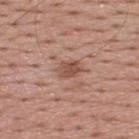The lesion was photographed on a routine skin check and not biopsied; there is no pathology result. A male subject roughly 55 years of age. Approximately 3 mm at its widest. The tile uses white-light illumination. An algorithmic analysis of the crop reported an area of roughly 4.5 mm². The analysis additionally found a mean CIELAB color near L≈51 a*≈22 b*≈27 and about 10 CIELAB-L* units darker than the surrounding skin. The software also gave a border-irregularity rating of about 2.5/10, a color-variation rating of about 2.5/10, and peripheral color asymmetry of about 1. Cropped from a total-body skin-imaging series; the visible field is about 15 mm. Located on the upper back.